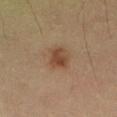Recorded during total-body skin imaging; not selected for excision or biopsy.
On the lower back.
This is a cross-polarized tile.
The patient is a male approximately 55 years of age.
A lesion tile, about 15 mm wide, cut from a 3D total-body photograph.